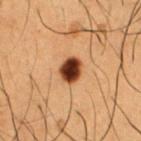biopsy status = no biopsy performed (imaged during a skin exam)
illumination = cross-polarized
imaging modality = ~15 mm crop, total-body skin-cancer survey
lesion size = about 3 mm
location = the chest
patient = male, in their 50s